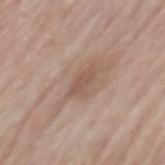Impression: Imaged during a routine full-body skin examination; the lesion was not biopsied and no histopathology is available.